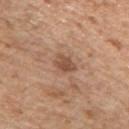Imaged during a routine full-body skin examination; the lesion was not biopsied and no histopathology is available. The patient is a male about 65 years old. Cropped from a whole-body photographic skin survey; the tile spans about 15 mm. The lesion is located on the arm.